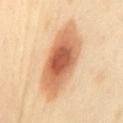<case>
  <biopsy_status>not biopsied; imaged during a skin examination</biopsy_status>
  <image>
    <source>total-body photography crop</source>
    <field_of_view_mm>15</field_of_view_mm>
  </image>
  <patient>
    <sex>female</sex>
    <age_approx>35</age_approx>
  </patient>
  <site>mid back</site>
  <lighting>cross-polarized</lighting>
  <lesion_size>
    <long_diameter_mm_approx>10.0</long_diameter_mm_approx>
  </lesion_size>
</case>